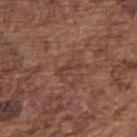notes: total-body-photography surveillance lesion; no biopsy | lighting: white-light | automated metrics: a shape eccentricity near 0.9 and a shape-asymmetry score of about 0.4 (0 = symmetric); a mean CIELAB color near L≈40 a*≈21 b*≈25, roughly 6 lightness units darker than nearby skin, and a normalized border contrast of about 5; a nevus-likeness score of about 0/100 and lesion-presence confidence of about 80/100 | acquisition: ~15 mm tile from a whole-body skin photo | site: the right upper arm | lesion diameter: ≈3 mm | patient: male, aged around 75.